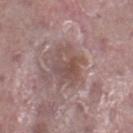Case summary:
- follow-up · imaged on a skin check; not biopsied
- acquisition · total-body-photography crop, ~15 mm field of view
- subject · male, roughly 40 years of age
- anatomic site · the right lower leg